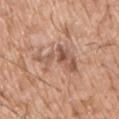Assessment:
Imaged during a routine full-body skin examination; the lesion was not biopsied and no histopathology is available.
Background:
This image is a 15 mm lesion crop taken from a total-body photograph. From the chest. A male patient, in their mid- to late 70s. Measured at roughly 7 mm in maximum diameter. Automated image analysis of the tile measured a lesion area of about 11 mm², an eccentricity of roughly 0.9, and a shape-asymmetry score of about 0.7 (0 = symmetric). The analysis additionally found a classifier nevus-likeness of about 0/100.